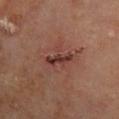Case summary:
• notes: catalogued during a skin exam; not biopsied
• lighting: cross-polarized
• size: ~3.5 mm (longest diameter)
• image: ~15 mm crop, total-body skin-cancer survey
• patient: male, aged 68–72
• site: the right lower leg